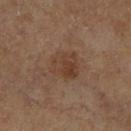No biopsy was performed on this lesion — it was imaged during a full skin examination and was not determined to be concerning.
The patient is a female aged around 60.
The recorded lesion diameter is about 3.5 mm.
The tile uses cross-polarized illumination.
From the right lower leg.
Cropped from a total-body skin-imaging series; the visible field is about 15 mm.